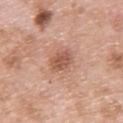No biopsy was performed on this lesion — it was imaged during a full skin examination and was not determined to be concerning.
From the upper back.
A roughly 15 mm field-of-view crop from a total-body skin photograph.
An algorithmic analysis of the crop reported a footprint of about 6 mm² and a shape eccentricity near 0.8. And it measured internal color variation of about 3 on a 0–10 scale and radial color variation of about 1.
A male subject, aged 48 to 52.
About 3.5 mm across.
This is a white-light tile.